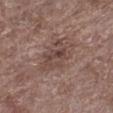biopsy_status: not biopsied; imaged during a skin examination
site: left lower leg
image:
  source: total-body photography crop
  field_of_view_mm: 15
lesion_size:
  long_diameter_mm_approx: 5.5
patient:
  sex: male
  age_approx: 70